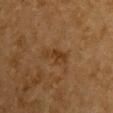<tbp_lesion>
  <biopsy_status>not biopsied; imaged during a skin examination</biopsy_status>
  <lighting>cross-polarized</lighting>
  <site>chest</site>
  <lesion_size>
    <long_diameter_mm_approx>3.5</long_diameter_mm_approx>
  </lesion_size>
  <image>
    <source>total-body photography crop</source>
    <field_of_view_mm>15</field_of_view_mm>
  </image>
  <patient>
    <sex>male</sex>
    <age_approx>85+</age_approx>
  </patient>
  <automated_metrics>
    <area_mm2_approx>4.5</area_mm2_approx>
    <eccentricity>0.9</eccentricity>
    <shape_asymmetry>0.35</shape_asymmetry>
    <nevus_likeness_0_100>0</nevus_likeness_0_100>
    <lesion_detection_confidence_0_100>100</lesion_detection_confidence_0_100>
  </automated_metrics>
</tbp_lesion>